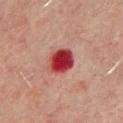The lesion was tiled from a total-body skin photograph and was not biopsied.
From the upper back.
A male subject, aged 68 to 72.
The total-body-photography lesion software estimated two-axis asymmetry of about 0.15. It also reported a mean CIELAB color near L≈34 a*≈35 b*≈24 and a normalized border contrast of about 13.5. And it measured border irregularity of about 1.5 on a 0–10 scale, internal color variation of about 5 on a 0–10 scale, and radial color variation of about 1.5.
A 15 mm close-up extracted from a 3D total-body photography capture.